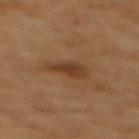<lesion>
  <biopsy_status>not biopsied; imaged during a skin examination</biopsy_status>
  <automated_metrics>
    <cielab_L>33</cielab_L>
    <cielab_a>17</cielab_a>
    <cielab_b>28</cielab_b>
    <vs_skin_darker_L>8.0</vs_skin_darker_L>
    <vs_skin_contrast_norm>7.5</vs_skin_contrast_norm>
    <border_irregularity_0_10>3.5</border_irregularity_0_10>
    <color_variation_0_10>2.5</color_variation_0_10>
    <peripheral_color_asymmetry>1.0</peripheral_color_asymmetry>
  </automated_metrics>
  <site>back</site>
  <lighting>cross-polarized</lighting>
  <lesion_size>
    <long_diameter_mm_approx>4.0</long_diameter_mm_approx>
  </lesion_size>
  <patient>
    <sex>female</sex>
    <age_approx>55</age_approx>
  </patient>
  <image>
    <source>total-body photography crop</source>
    <field_of_view_mm>15</field_of_view_mm>
  </image>
</lesion>